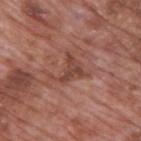Q: Was a biopsy performed?
A: imaged on a skin check; not biopsied
Q: Lesion size?
A: ≈4.5 mm
Q: Where on the body is the lesion?
A: the upper back
Q: Patient demographics?
A: male, in their 70s
Q: How was this image acquired?
A: 15 mm crop, total-body photography
Q: Automated lesion metrics?
A: a border-irregularity rating of about 8.5/10, internal color variation of about 2.5 on a 0–10 scale, and a peripheral color-asymmetry measure near 1